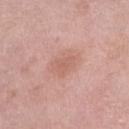Q: Was this lesion biopsied?
A: total-body-photography surveillance lesion; no biopsy
Q: What kind of image is this?
A: 15 mm crop, total-body photography
Q: Where on the body is the lesion?
A: the right lower leg
Q: Who is the patient?
A: female, aged 68–72